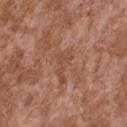notes — total-body-photography surveillance lesion; no biopsy | tile lighting — white-light illumination | subject — male, aged 43–47 | diameter — about 4 mm | image — total-body-photography crop, ~15 mm field of view | anatomic site — the upper back.